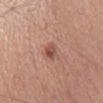No biopsy was performed on this lesion — it was imaged during a full skin examination and was not determined to be concerning.
The lesion's longest dimension is about 3 mm.
Imaged with white-light lighting.
Automated image analysis of the tile measured a lesion area of about 3.5 mm² and an eccentricity of roughly 0.8.
A region of skin cropped from a whole-body photographic capture, roughly 15 mm wide.
From the chest.
A female patient about 45 years old.